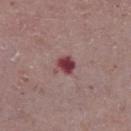Captured during whole-body skin photography for melanoma surveillance; the lesion was not biopsied. This image is a 15 mm lesion crop taken from a total-body photograph. Longest diameter approximately 2.5 mm. A male patient aged approximately 70. Located on the chest. Automated tile analysis of the lesion measured an outline eccentricity of about 0.5 (0 = round, 1 = elongated) and a shape-asymmetry score of about 0.3 (0 = symmetric). And it measured a border-irregularity rating of about 3/10 and a peripheral color-asymmetry measure near 1. The software also gave a nevus-likeness score of about 0/100 and lesion-presence confidence of about 100/100.